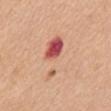  biopsy_status: not biopsied; imaged during a skin examination
  automated_metrics:
    cielab_L: 62
    cielab_a: 25
    cielab_b: 30
    vs_skin_darker_L: 9.0
    vs_skin_contrast_norm: 6.0
  lesion_size:
    long_diameter_mm_approx: 6.0
  patient:
    sex: female
    age_approx: 55
  lighting: white-light
  site: mid back
  image:
    source: total-body photography crop
    field_of_view_mm: 15